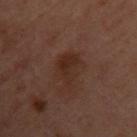A female patient aged approximately 55. A 15 mm crop from a total-body photograph taken for skin-cancer surveillance. The lesion is on the upper back.